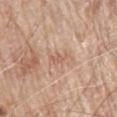Clinical impression: This lesion was catalogued during total-body skin photography and was not selected for biopsy. Acquisition and patient details: On the mid back. Imaged with white-light lighting. A male subject, roughly 80 years of age. Cropped from a whole-body photographic skin survey; the tile spans about 15 mm. An algorithmic analysis of the crop reported a border-irregularity rating of about 5.5/10, internal color variation of about 0.5 on a 0–10 scale, and a peripheral color-asymmetry measure near 0.5. The recorded lesion diameter is about 3 mm.